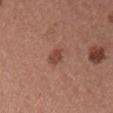Q: Was this lesion biopsied?
A: imaged on a skin check; not biopsied
Q: What did automated image analysis measure?
A: a footprint of about 3.5 mm², a shape eccentricity near 0.8, and a symmetry-axis asymmetry near 0.25; a border-irregularity rating of about 2.5/10 and peripheral color asymmetry of about 0.5
Q: What is the imaging modality?
A: ~15 mm tile from a whole-body skin photo
Q: What is the anatomic site?
A: the chest
Q: Who is the patient?
A: male, about 40 years old